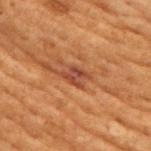{
  "biopsy_status": "not biopsied; imaged during a skin examination",
  "lighting": "cross-polarized",
  "image": {
    "source": "total-body photography crop",
    "field_of_view_mm": 15
  },
  "patient": {
    "sex": "female",
    "age_approx": 80
  },
  "lesion_size": {
    "long_diameter_mm_approx": 3.5
  },
  "automated_metrics": {
    "area_mm2_approx": 5.5,
    "eccentricity": 0.75,
    "shape_asymmetry": 0.3,
    "vs_skin_darker_L": 7.0
  },
  "site": "chest"
}